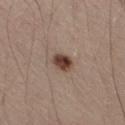Case summary:
• notes — catalogued during a skin exam; not biopsied
• anatomic site — the right thigh
• image — total-body-photography crop, ~15 mm field of view
• diameter — ≈2.5 mm
• subject — male, about 55 years old